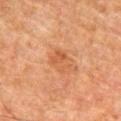biopsy status: no biopsy performed (imaged during a skin exam) | patient: male, aged 78 to 82 | anatomic site: the abdomen | acquisition: ~15 mm crop, total-body skin-cancer survey | TBP lesion metrics: a lesion color around L≈44 a*≈22 b*≈33 in CIELAB, a lesion–skin lightness drop of about 6, and a normalized lesion–skin contrast near 5.5; a nevus-likeness score of about 5/100 and a lesion-detection confidence of about 100/100 | illumination: cross-polarized | size: ≈2.5 mm.